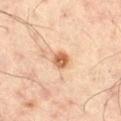{"site": "right thigh", "image": {"source": "total-body photography crop", "field_of_view_mm": 15}, "patient": {"sex": "male", "age_approx": 45}, "automated_metrics": {"nevus_likeness_0_100": 90, "lesion_detection_confidence_0_100": 100}, "lighting": "cross-polarized", "lesion_size": {"long_diameter_mm_approx": 2.5}}